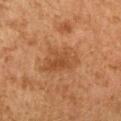Notes:
• biopsy status · total-body-photography surveillance lesion; no biopsy
• image source · total-body-photography crop, ~15 mm field of view
• lighting · cross-polarized
• image-analysis metrics · a shape eccentricity near 0.75 and two-axis asymmetry of about 0.3; a mean CIELAB color near L≈43 a*≈21 b*≈34, a lesion–skin lightness drop of about 7, and a lesion-to-skin contrast of about 6 (normalized; higher = more distinct); a peripheral color-asymmetry measure near 1.5
• anatomic site · the left upper arm
• patient · female, about 60 years old
• diameter · ~5 mm (longest diameter)